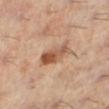Q: Where on the body is the lesion?
A: the left lower leg
Q: Patient demographics?
A: female, aged 58–62
Q: How was this image acquired?
A: 15 mm crop, total-body photography